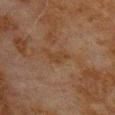workup: total-body-photography surveillance lesion; no biopsy | lesion size: about 2.5 mm | TBP lesion metrics: an outline eccentricity of about 0.75 (0 = round, 1 = elongated) and a symmetry-axis asymmetry near 0.4; an average lesion color of about L≈31 a*≈15 b*≈26 (CIELAB) and a lesion–skin lightness drop of about 4 | illumination: cross-polarized illumination | subject: male, roughly 80 years of age | location: the upper back | image source: total-body-photography crop, ~15 mm field of view.